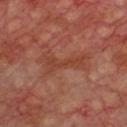biopsy_status: not biopsied; imaged during a skin examination
automated_metrics:
  area_mm2_approx: 7.0
  shape_asymmetry: 0.5
  border_irregularity_0_10: 7.0
  peripheral_color_asymmetry: 0.5
image:
  source: total-body photography crop
  field_of_view_mm: 15
site: front of the torso
lesion_size:
  long_diameter_mm_approx: 5.0
patient:
  sex: male
  age_approx: 70